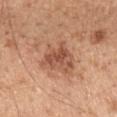{"biopsy_status": "not biopsied; imaged during a skin examination", "lesion_size": {"long_diameter_mm_approx": 4.5}, "patient": {"sex": "male", "age_approx": 45}, "site": "mid back", "image": {"source": "total-body photography crop", "field_of_view_mm": 15}, "lighting": "white-light"}